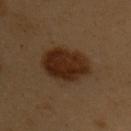This lesion was catalogued during total-body skin photography and was not selected for biopsy. Measured at roughly 6.5 mm in maximum diameter. Located on the back. The patient is a female approximately 60 years of age. This is a cross-polarized tile. The lesion-visualizer software estimated a shape eccentricity near 0.85 and a shape-asymmetry score of about 0.1 (0 = symmetric). The analysis additionally found an automated nevus-likeness rating near 100 out of 100 and a lesion-detection confidence of about 100/100. A close-up tile cropped from a whole-body skin photograph, about 15 mm across.